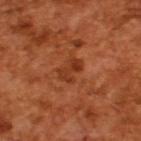Assessment:
Recorded during total-body skin imaging; not selected for excision or biopsy.
Image and clinical context:
The patient is a male approximately 65 years of age. A close-up tile cropped from a whole-body skin photograph, about 15 mm across. Automated image analysis of the tile measured a border-irregularity index near 4.5/10, a color-variation rating of about 3.5/10, and radial color variation of about 1.5. The lesion's longest dimension is about 3 mm.